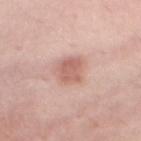No biopsy was performed on this lesion — it was imaged during a full skin examination and was not determined to be concerning. A female subject, approximately 35 years of age. The lesion is located on the chest. This image is a 15 mm lesion crop taken from a total-body photograph.